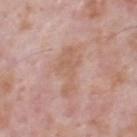Q: Was this lesion biopsied?
A: total-body-photography surveillance lesion; no biopsy
Q: Patient demographics?
A: male, aged approximately 70
Q: What is the imaging modality?
A: total-body-photography crop, ~15 mm field of view
Q: Automated lesion metrics?
A: a lesion area of about 12 mm², an outline eccentricity of about 0.85 (0 = round, 1 = elongated), and a shape-asymmetry score of about 0.5 (0 = symmetric)
Q: What is the lesion's diameter?
A: about 5.5 mm
Q: Lesion location?
A: the head or neck